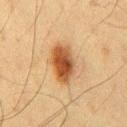Context: The subject is a male aged approximately 60. This image is a 15 mm lesion crop taken from a total-body photograph. About 5 mm across. The lesion-visualizer software estimated a lesion area of about 11 mm², an outline eccentricity of about 0.85 (0 = round, 1 = elongated), and a shape-asymmetry score of about 0.2 (0 = symmetric). This is a cross-polarized tile. The lesion is on the chest.